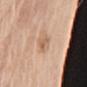This lesion was catalogued during total-body skin photography and was not selected for biopsy.
A close-up tile cropped from a whole-body skin photograph, about 15 mm across.
A female patient aged approximately 75.
Located on the left upper arm.